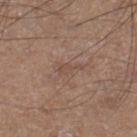patient = male, aged approximately 60; imaging modality = 15 mm crop, total-body photography; location = the right lower leg; tile lighting = white-light.